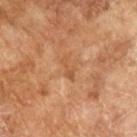Imaged during a routine full-body skin examination; the lesion was not biopsied and no histopathology is available. The recorded lesion diameter is about 3 mm. Automated tile analysis of the lesion measured a lesion color around L≈53 a*≈23 b*≈37 in CIELAB and about 7 CIELAB-L* units darker than the surrounding skin. And it measured a border-irregularity index near 6.5/10 and internal color variation of about 0 on a 0–10 scale. The subject is a male in their mid- to late 60s. This image is a 15 mm lesion crop taken from a total-body photograph.